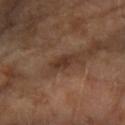| key | value |
|---|---|
| imaging modality | ~15 mm tile from a whole-body skin photo |
| diameter | ~3 mm (longest diameter) |
| lighting | cross-polarized illumination |
| anatomic site | the right forearm |
| subject | female, roughly 70 years of age |